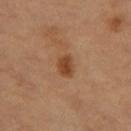biopsy status — total-body-photography surveillance lesion; no biopsy | diameter — ≈3 mm | image-analysis metrics — a lesion area of about 5 mm² and an outline eccentricity of about 0.7 (0 = round, 1 = elongated); a nevus-likeness score of about 90/100 and a detector confidence of about 100 out of 100 that the crop contains a lesion | image — total-body-photography crop, ~15 mm field of view | tile lighting — cross-polarized illumination | location — the left thigh | subject — female, in their 60s.